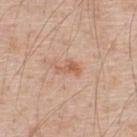Q: Was this lesion biopsied?
A: no biopsy performed (imaged during a skin exam)
Q: What did automated image analysis measure?
A: a footprint of about 4 mm², an eccentricity of roughly 0.85, and a shape-asymmetry score of about 0.5 (0 = symmetric); a mean CIELAB color near L≈60 a*≈22 b*≈33; a border-irregularity rating of about 6/10, a color-variation rating of about 2/10, and peripheral color asymmetry of about 0.5; a nevus-likeness score of about 40/100 and lesion-presence confidence of about 100/100
Q: How large is the lesion?
A: about 3.5 mm
Q: How was the tile lit?
A: white-light
Q: What is the anatomic site?
A: the upper back
Q: What are the patient's age and sex?
A: male, roughly 50 years of age
Q: What is the imaging modality?
A: ~15 mm crop, total-body skin-cancer survey